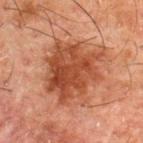Image and clinical context: The patient is a male in their 50s. A region of skin cropped from a whole-body photographic capture, roughly 15 mm wide. About 7 mm across. The lesion is located on the upper back. Automated tile analysis of the lesion measured a lesion–skin lightness drop of about 10 and a normalized lesion–skin contrast near 9. It also reported a border-irregularity index near 4.5/10 and a color-variation rating of about 4.5/10.